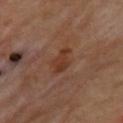Impression:
The lesion was photographed on a routine skin check and not biopsied; there is no pathology result.
Image and clinical context:
A male patient, in their 70s. From the upper back. The recorded lesion diameter is about 3.5 mm. A lesion tile, about 15 mm wide, cut from a 3D total-body photograph. Imaged with cross-polarized lighting. The total-body-photography lesion software estimated a lesion area of about 6 mm² and an eccentricity of roughly 0.8. The analysis additionally found a lesion–skin lightness drop of about 7 and a normalized lesion–skin contrast near 7.